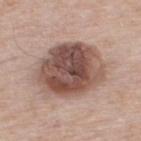Q: Was a biopsy performed?
A: imaged on a skin check; not biopsied
Q: How large is the lesion?
A: ~7.5 mm (longest diameter)
Q: Automated lesion metrics?
A: an area of roughly 35 mm², a shape eccentricity near 0.55, and a symmetry-axis asymmetry near 0.1; an average lesion color of about L≈50 a*≈19 b*≈24 (CIELAB), roughly 16 lightness units darker than nearby skin, and a normalized lesion–skin contrast near 11; a border-irregularity index near 1/10, internal color variation of about 8.5 on a 0–10 scale, and radial color variation of about 2.5; a nevus-likeness score of about 30/100 and lesion-presence confidence of about 100/100
Q: Where on the body is the lesion?
A: the upper back
Q: What is the imaging modality?
A: ~15 mm crop, total-body skin-cancer survey
Q: How was the tile lit?
A: white-light
Q: What are the patient's age and sex?
A: male, about 55 years old